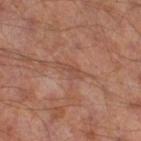follow-up: total-body-photography surveillance lesion; no biopsy | illumination: cross-polarized illumination | patient: male, aged approximately 65 | body site: the right thigh | diameter: ~3 mm (longest diameter) | image source: ~15 mm tile from a whole-body skin photo | automated metrics: an average lesion color of about L≈45 a*≈21 b*≈27 (CIELAB), roughly 6 lightness units darker than nearby skin, and a lesion-to-skin contrast of about 5 (normalized; higher = more distinct); an automated nevus-likeness rating near 0 out of 100 and a detector confidence of about 80 out of 100 that the crop contains a lesion.